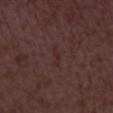biopsy status = imaged on a skin check; not biopsied | size = about 3 mm | lighting = white-light illumination | image = 15 mm crop, total-body photography | patient = female, about 35 years old | location = the left thigh.